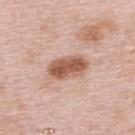The lesion was photographed on a routine skin check and not biopsied; there is no pathology result. A female subject, approximately 65 years of age. The tile uses white-light illumination. The lesion is on the upper back. Approximately 4.5 mm at its widest. A 15 mm close-up tile from a total-body photography series done for melanoma screening.